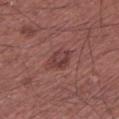Captured during whole-body skin photography for melanoma surveillance; the lesion was not biopsied.